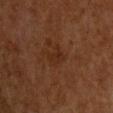Q: Was this lesion biopsied?
A: total-body-photography surveillance lesion; no biopsy
Q: Lesion size?
A: ≈3 mm
Q: Automated lesion metrics?
A: a lesion area of about 3.5 mm², an eccentricity of roughly 0.85, and a symmetry-axis asymmetry near 0.35; a mean CIELAB color near L≈23 a*≈18 b*≈26, roughly 4 lightness units darker than nearby skin, and a lesion-to-skin contrast of about 5 (normalized; higher = more distinct); an automated nevus-likeness rating near 0 out of 100 and a detector confidence of about 100 out of 100 that the crop contains a lesion
Q: Who is the patient?
A: male, aged around 65
Q: What is the imaging modality?
A: total-body-photography crop, ~15 mm field of view
Q: How was the tile lit?
A: cross-polarized illumination
Q: What is the anatomic site?
A: the back